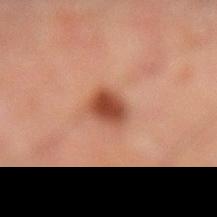biopsy_status: not biopsied; imaged during a skin examination
image:
  source: total-body photography crop
  field_of_view_mm: 15
site: left lower leg
patient:
  sex: male
  age_approx: 65